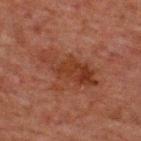Notes:
* biopsy status — imaged on a skin check; not biopsied
* image source — 15 mm crop, total-body photography
* subject — male, aged approximately 60
* site — the upper back
* lesion size — ~7 mm (longest diameter)
* illumination — cross-polarized illumination